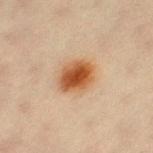<record>
  <biopsy_status>not biopsied; imaged during a skin examination</biopsy_status>
  <automated_metrics>
    <area_mm2_approx>9.5</area_mm2_approx>
    <eccentricity>0.6</eccentricity>
    <shape_asymmetry>0.15</shape_asymmetry>
    <nevus_likeness_0_100>100</nevus_likeness_0_100>
    <lesion_detection_confidence_0_100>100</lesion_detection_confidence_0_100>
  </automated_metrics>
  <lesion_size>
    <long_diameter_mm_approx>4.0</long_diameter_mm_approx>
  </lesion_size>
  <lighting>cross-polarized</lighting>
  <image>
    <source>total-body photography crop</source>
    <field_of_view_mm>15</field_of_view_mm>
  </image>
  <site>left thigh</site>
  <patient>
    <sex>female</sex>
    <age_approx>45</age_approx>
  </patient>
</record>